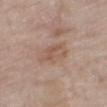  biopsy_status: not biopsied; imaged during a skin examination
  lesion_size:
    long_diameter_mm_approx: 4.5
  site: right thigh
  image:
    source: total-body photography crop
    field_of_view_mm: 15
  automated_metrics:
    area_mm2_approx: 8.5
    shape_asymmetry: 0.15
    cielab_L: 55
    cielab_a: 17
    cielab_b: 27
    vs_skin_darker_L: 7.0
    vs_skin_contrast_norm: 5.5
    border_irregularity_0_10: 2.0
    color_variation_0_10: 4.0
    peripheral_color_asymmetry: 1.5
    nevus_likeness_0_100: 0
    lesion_detection_confidence_0_100: 100
  patient:
    sex: male
    age_approx: 80